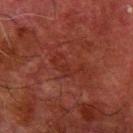This lesion was catalogued during total-body skin photography and was not selected for biopsy. The tile uses cross-polarized illumination. A 15 mm crop from a total-body photograph taken for skin-cancer surveillance. The lesion is on the arm. The patient is a male in their 80s.